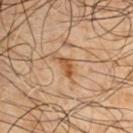The patient is a male aged 48 to 52. The lesion is located on the left upper arm. A lesion tile, about 15 mm wide, cut from a 3D total-body photograph. Approximately 2.5 mm at its widest.